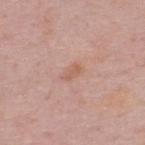  biopsy_status: not biopsied; imaged during a skin examination